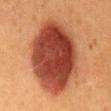A close-up tile cropped from a whole-body skin photograph, about 15 mm across.
The subject is a female about 50 years old.
From the mid back.
Imaged with cross-polarized lighting.